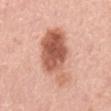notes — no biopsy performed (imaged during a skin exam) | image source — total-body-photography crop, ~15 mm field of view | size — ≈8 mm | subject — male, aged 63 to 67 | automated metrics — an area of roughly 23 mm² and an eccentricity of roughly 0.85; a mean CIELAB color near L≈59 a*≈26 b*≈31, roughly 16 lightness units darker than nearby skin, and a normalized lesion–skin contrast near 9.5; border irregularity of about 4 on a 0–10 scale and radial color variation of about 2.5; a classifier nevus-likeness of about 90/100 and lesion-presence confidence of about 100/100 | lighting — white-light | anatomic site — the mid back.